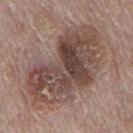follow-up — catalogued during a skin exam; not biopsied | site — the mid back | TBP lesion metrics — an outline eccentricity of about 0.85 (0 = round, 1 = elongated); a mean CIELAB color near L≈46 a*≈16 b*≈21; a classifier nevus-likeness of about 0/100 and a detector confidence of about 100 out of 100 that the crop contains a lesion | image source — ~15 mm crop, total-body skin-cancer survey | lesion size — about 11 mm | patient — male, aged 68 to 72.